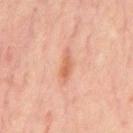Q: Is there a histopathology result?
A: catalogued during a skin exam; not biopsied
Q: Who is the patient?
A: male, aged 63–67
Q: Lesion location?
A: the chest
Q: What lighting was used for the tile?
A: cross-polarized
Q: Lesion size?
A: ≈3.5 mm
Q: What is the imaging modality?
A: 15 mm crop, total-body photography